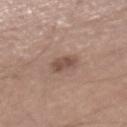notes=total-body-photography surveillance lesion; no biopsy | lesion size=≈2.5 mm | patient=male, roughly 40 years of age | body site=the right lower leg | illumination=white-light illumination | imaging modality=total-body-photography crop, ~15 mm field of view | TBP lesion metrics=a lesion area of about 4.5 mm² and two-axis asymmetry of about 0.25; an average lesion color of about L≈49 a*≈18 b*≈24 (CIELAB), roughly 10 lightness units darker than nearby skin, and a normalized border contrast of about 7.5.